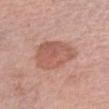  biopsy_status: not biopsied; imaged during a skin examination
  image:
    source: total-body photography crop
    field_of_view_mm: 15
  patient:
    sex: female
    age_approx: 65
  site: right forearm